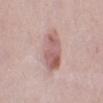Clinical impression: The lesion was photographed on a routine skin check and not biopsied; there is no pathology result. Context: A lesion tile, about 15 mm wide, cut from a 3D total-body photograph. Measured at roughly 5 mm in maximum diameter. Captured under white-light illumination. A female patient, approximately 40 years of age. Located on the left lower leg.